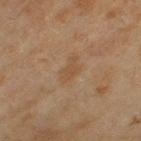<record>
  <biopsy_status>not biopsied; imaged during a skin examination</biopsy_status>
  <lesion_size>
    <long_diameter_mm_approx>3.0</long_diameter_mm_approx>
  </lesion_size>
  <site>left thigh</site>
  <patient>
    <sex>female</sex>
    <age_approx>60</age_approx>
  </patient>
  <lighting>cross-polarized</lighting>
  <image>
    <source>total-body photography crop</source>
    <field_of_view_mm>15</field_of_view_mm>
  </image>
</record>